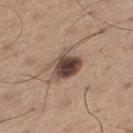imaging modality = total-body-photography crop, ~15 mm field of view; illumination = white-light; lesion size = ~3.5 mm (longest diameter); subject = male, in their mid-60s; site = the right thigh.